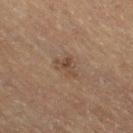This lesion was catalogued during total-body skin photography and was not selected for biopsy. A female patient aged 53–57. An algorithmic analysis of the crop reported a mean CIELAB color near L≈39 a*≈15 b*≈25 and a lesion–skin lightness drop of about 7. The analysis additionally found a border-irregularity rating of about 6/10, internal color variation of about 2.5 on a 0–10 scale, and radial color variation of about 0.5. The tile uses cross-polarized illumination. From the right thigh. Cropped from a total-body skin-imaging series; the visible field is about 15 mm.